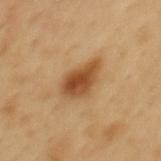Assessment:
Captured during whole-body skin photography for melanoma surveillance; the lesion was not biopsied.
Image and clinical context:
This image is a 15 mm lesion crop taken from a total-body photograph. A male subject, in their 50s. Located on the back. The lesion's longest dimension is about 4.5 mm. Automated image analysis of the tile measured a footprint of about 9.5 mm², a shape eccentricity near 0.8, and a symmetry-axis asymmetry near 0.3. And it measured a mean CIELAB color near L≈49 a*≈22 b*≈39, a lesion–skin lightness drop of about 13, and a normalized lesion–skin contrast near 9.5. It also reported a nevus-likeness score of about 95/100 and a detector confidence of about 100 out of 100 that the crop contains a lesion. Captured under cross-polarized illumination.